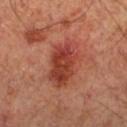Clinical impression:
Part of a total-body skin-imaging series; this lesion was reviewed on a skin check and was not flagged for biopsy.
Context:
An algorithmic analysis of the crop reported an area of roughly 17 mm² and a shape eccentricity near 0.8. And it measured a mean CIELAB color near L≈42 a*≈31 b*≈31 and a normalized lesion–skin contrast near 8.5. And it measured border irregularity of about 2 on a 0–10 scale and a color-variation rating of about 6/10. The analysis additionally found a detector confidence of about 100 out of 100 that the crop contains a lesion. The lesion's longest dimension is about 6 mm. A male subject, aged 63 to 67. Located on the right lower leg. Captured under cross-polarized illumination. A 15 mm crop from a total-body photograph taken for skin-cancer surveillance.